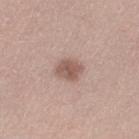Imaged during a routine full-body skin examination; the lesion was not biopsied and no histopathology is available.
A close-up tile cropped from a whole-body skin photograph, about 15 mm across.
Located on the left thigh.
The subject is a female about 45 years old.
The recorded lesion diameter is about 3 mm.